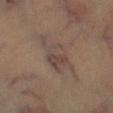Assessment:
No biopsy was performed on this lesion — it was imaged during a full skin examination and was not determined to be concerning.
Context:
This is a cross-polarized tile. A region of skin cropped from a whole-body photographic capture, roughly 15 mm wide. The lesion's longest dimension is about 6.5 mm. The lesion is located on the right lower leg. The patient is a male in their mid- to late 40s.